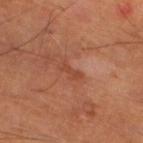Findings:
• illumination · cross-polarized illumination
• image · ~15 mm tile from a whole-body skin photo
• subject · male, aged approximately 65
• body site · the right lower leg
• automated lesion analysis · a lesion area of about 2 mm², an eccentricity of roughly 0.95, and a shape-asymmetry score of about 0.5 (0 = symmetric); a classifier nevus-likeness of about 0/100 and lesion-presence confidence of about 90/100